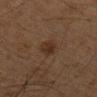| key | value |
|---|---|
| biopsy status | catalogued during a skin exam; not biopsied |